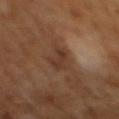Q: What lighting was used for the tile?
A: cross-polarized illumination
Q: What kind of image is this?
A: total-body-photography crop, ~15 mm field of view
Q: What did automated image analysis measure?
A: a footprint of about 5.5 mm², an outline eccentricity of about 0.9 (0 = round, 1 = elongated), and two-axis asymmetry of about 0.4; a lesion color around L≈34 a*≈18 b*≈27 in CIELAB, roughly 7 lightness units darker than nearby skin, and a normalized lesion–skin contrast near 7
Q: Patient demographics?
A: male, approximately 65 years of age
Q: What is the lesion's diameter?
A: about 4 mm